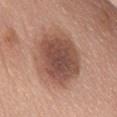The lesion is on the front of the torso. Measured at roughly 8 mm in maximum diameter. This is a white-light tile. The subject is a female roughly 60 years of age. A lesion tile, about 15 mm wide, cut from a 3D total-body photograph. Automated image analysis of the tile measured roughly 13 lightness units darker than nearby skin and a normalized lesion–skin contrast near 9. It also reported a border-irregularity index near 1.5/10, a color-variation rating of about 5/10, and peripheral color asymmetry of about 1.5. It also reported a nevus-likeness score of about 25/100 and a lesion-detection confidence of about 100/100.